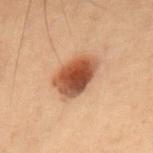* biopsy status · catalogued during a skin exam; not biopsied
* acquisition · 15 mm crop, total-body photography
* automated lesion analysis · a lesion color around L≈42 a*≈21 b*≈29 in CIELAB, about 15 CIELAB-L* units darker than the surrounding skin, and a normalized lesion–skin contrast near 11.5; a border-irregularity rating of about 2/10 and radial color variation of about 1.5; a nevus-likeness score of about 100/100 and a detector confidence of about 100 out of 100 that the crop contains a lesion
* lesion size · about 5 mm
* subject · male, about 55 years old
* anatomic site · the upper back
* tile lighting · cross-polarized